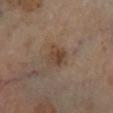Clinical impression: Captured during whole-body skin photography for melanoma surveillance; the lesion was not biopsied. Acquisition and patient details: A male patient aged approximately 70. Approximately 3 mm at its widest. The total-body-photography lesion software estimated an area of roughly 6.5 mm², an outline eccentricity of about 0.5 (0 = round, 1 = elongated), and a symmetry-axis asymmetry near 0.2. And it measured a lesion color around L≈40 a*≈15 b*≈26 in CIELAB, about 7 CIELAB-L* units darker than the surrounding skin, and a normalized border contrast of about 6.5. The analysis additionally found border irregularity of about 2.5 on a 0–10 scale. The software also gave a nevus-likeness score of about 15/100 and a detector confidence of about 100 out of 100 that the crop contains a lesion. A close-up tile cropped from a whole-body skin photograph, about 15 mm across. Located on the right lower leg.